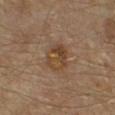Assessment: Recorded during total-body skin imaging; not selected for excision or biopsy. Image and clinical context: Longest diameter approximately 3.5 mm. Located on the right lower leg. A patient aged 53–57. Imaged with cross-polarized lighting. A region of skin cropped from a whole-body photographic capture, roughly 15 mm wide.